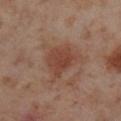notes: total-body-photography surveillance lesion; no biopsy
imaging modality: total-body-photography crop, ~15 mm field of view
patient: female, aged around 55
lesion diameter: ≈3.5 mm
location: the leg
lighting: cross-polarized